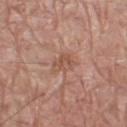The lesion was photographed on a routine skin check and not biopsied; there is no pathology result.